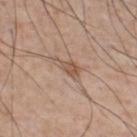<lesion>
  <biopsy_status>not biopsied; imaged during a skin examination</biopsy_status>
  <automated_metrics>
    <area_mm2_approx>5.0</area_mm2_approx>
    <eccentricity>0.9</eccentricity>
    <shape_asymmetry>0.45</shape_asymmetry>
    <cielab_L>54</cielab_L>
    <cielab_a>17</cielab_a>
    <cielab_b>28</cielab_b>
    <vs_skin_darker_L>9.0</vs_skin_darker_L>
    <vs_skin_contrast_norm>6.5</vs_skin_contrast_norm>
    <color_variation_0_10>2.5</color_variation_0_10>
    <peripheral_color_asymmetry>1.0</peripheral_color_asymmetry>
    <nevus_likeness_0_100>35</nevus_likeness_0_100>
    <lesion_detection_confidence_0_100>100</lesion_detection_confidence_0_100>
  </automated_metrics>
  <lighting>white-light</lighting>
  <lesion_size>
    <long_diameter_mm_approx>4.0</long_diameter_mm_approx>
  </lesion_size>
  <image>
    <source>total-body photography crop</source>
    <field_of_view_mm>15</field_of_view_mm>
  </image>
  <patient>
    <sex>male</sex>
    <age_approx>50</age_approx>
  </patient>
  <site>chest</site>
</lesion>